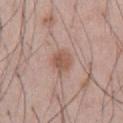Q: Was this lesion biopsied?
A: catalogued during a skin exam; not biopsied
Q: Automated lesion metrics?
A: an area of roughly 6 mm² and a symmetry-axis asymmetry near 0.15; an average lesion color of about L≈55 a*≈19 b*≈27 (CIELAB), roughly 9 lightness units darker than nearby skin, and a lesion-to-skin contrast of about 7 (normalized; higher = more distinct); border irregularity of about 1.5 on a 0–10 scale, a color-variation rating of about 4/10, and peripheral color asymmetry of about 1.5
Q: What lighting was used for the tile?
A: white-light illumination
Q: What is the imaging modality?
A: ~15 mm crop, total-body skin-cancer survey
Q: What is the lesion's diameter?
A: ~3 mm (longest diameter)
Q: What are the patient's age and sex?
A: male, about 55 years old
Q: Lesion location?
A: the abdomen